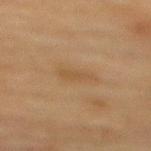follow-up = imaged on a skin check; not biopsied.